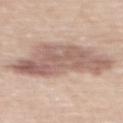This lesion was catalogued during total-body skin photography and was not selected for biopsy.
The tile uses white-light illumination.
On the upper back.
Cropped from a total-body skin-imaging series; the visible field is about 15 mm.
Measured at roughly 10 mm in maximum diameter.
The total-body-photography lesion software estimated a within-lesion color-variation index near 5/10 and radial color variation of about 2.
The subject is a male in their 70s.